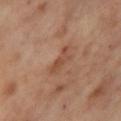No biopsy was performed on this lesion — it was imaged during a full skin examination and was not determined to be concerning. The total-body-photography lesion software estimated an area of roughly 3.5 mm², an eccentricity of roughly 0.9, and two-axis asymmetry of about 0.45. And it measured a lesion–skin lightness drop of about 8 and a lesion-to-skin contrast of about 6 (normalized; higher = more distinct). The software also gave a classifier nevus-likeness of about 0/100 and lesion-presence confidence of about 100/100. A roughly 15 mm field-of-view crop from a total-body skin photograph. The subject is a female in their mid- to late 50s. The lesion is located on the right thigh.